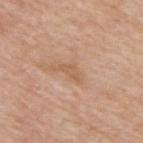This lesion was catalogued during total-body skin photography and was not selected for biopsy. The patient is a male aged around 80. A region of skin cropped from a whole-body photographic capture, roughly 15 mm wide. On the upper back.